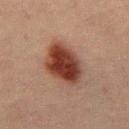| field | value |
|---|---|
| notes | no biopsy performed (imaged during a skin exam) |
| illumination | cross-polarized illumination |
| body site | the mid back |
| automated metrics | a shape eccentricity near 0.6 and two-axis asymmetry of about 0.15; a mean CIELAB color near L≈30 a*≈20 b*≈23, a lesion–skin lightness drop of about 14, and a normalized lesion–skin contrast near 12.5; a border-irregularity rating of about 1.5/10 and a color-variation rating of about 4.5/10; a classifier nevus-likeness of about 100/100 and a detector confidence of about 100 out of 100 that the crop contains a lesion |
| image | ~15 mm crop, total-body skin-cancer survey |
| diameter | ~5.5 mm (longest diameter) |
| patient | male, in their 50s |